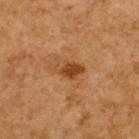Clinical impression: Part of a total-body skin-imaging series; this lesion was reviewed on a skin check and was not flagged for biopsy. Context: The lesion's longest dimension is about 3.5 mm. Automated image analysis of the tile measured a lesion area of about 5.5 mm² and two-axis asymmetry of about 0.35. The analysis additionally found a lesion color around L≈41 a*≈23 b*≈36 in CIELAB and roughly 10 lightness units darker than nearby skin. The software also gave a border-irregularity index near 4/10 and a peripheral color-asymmetry measure near 0.5. The software also gave a nevus-likeness score of about 80/100 and a detector confidence of about 100 out of 100 that the crop contains a lesion. A male subject aged approximately 60. The tile uses cross-polarized illumination. This image is a 15 mm lesion crop taken from a total-body photograph. On the back.